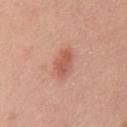| key | value |
|---|---|
| TBP lesion metrics | an average lesion color of about L≈57 a*≈26 b*≈30 (CIELAB) and a normalized border contrast of about 7; an automated nevus-likeness rating near 95 out of 100 and lesion-presence confidence of about 100/100 |
| patient | male, aged approximately 40 |
| imaging modality | ~15 mm tile from a whole-body skin photo |
| body site | the chest |
| lighting | white-light |
| diameter | ≈3.5 mm |